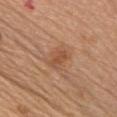The lesion was photographed on a routine skin check and not biopsied; there is no pathology result. The total-body-photography lesion software estimated a lesion area of about 3.5 mm², a shape eccentricity near 0.85, and a symmetry-axis asymmetry near 0.25. The software also gave a mean CIELAB color near L≈51 a*≈23 b*≈34, a lesion–skin lightness drop of about 8, and a normalized border contrast of about 6. It also reported a border-irregularity index near 2.5/10, internal color variation of about 1.5 on a 0–10 scale, and a peripheral color-asymmetry measure near 0.5. The subject is a female approximately 60 years of age. From the chest. A lesion tile, about 15 mm wide, cut from a 3D total-body photograph.